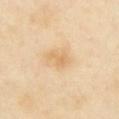notes — total-body-photography surveillance lesion; no biopsy | size — ≈3 mm | image source — total-body-photography crop, ~15 mm field of view | tile lighting — cross-polarized illumination | subject — female, roughly 40 years of age | location — the chest.